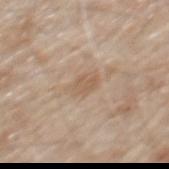This lesion was catalogued during total-body skin photography and was not selected for biopsy.
The total-body-photography lesion software estimated an area of roughly 5.5 mm², an outline eccentricity of about 0.75 (0 = round, 1 = elongated), and two-axis asymmetry of about 0.25. It also reported a border-irregularity rating of about 2.5/10, a color-variation rating of about 2/10, and a peripheral color-asymmetry measure near 1. The software also gave a classifier nevus-likeness of about 0/100.
Longest diameter approximately 3 mm.
The patient is a male aged 78 to 82.
On the mid back.
This is a white-light tile.
A region of skin cropped from a whole-body photographic capture, roughly 15 mm wide.